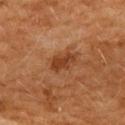• workup · no biopsy performed (imaged during a skin exam)